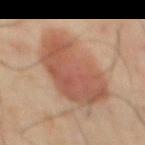Captured during whole-body skin photography for melanoma surveillance; the lesion was not biopsied. A close-up tile cropped from a whole-body skin photograph, about 15 mm across. Measured at roughly 8.5 mm in maximum diameter. On the abdomen. A male subject, roughly 70 years of age. The tile uses cross-polarized illumination.